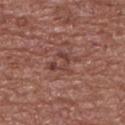No biopsy was performed on this lesion — it was imaged during a full skin examination and was not determined to be concerning. On the upper back. Cropped from a whole-body photographic skin survey; the tile spans about 15 mm. A male subject aged 53 to 57. About 3.5 mm across.